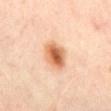Part of a total-body skin-imaging series; this lesion was reviewed on a skin check and was not flagged for biopsy. The patient is a male aged 63–67. The lesion is on the abdomen. This image is a 15 mm lesion crop taken from a total-body photograph. Longest diameter approximately 4 mm. An algorithmic analysis of the crop reported a mean CIELAB color near L≈64 a*≈24 b*≈37, roughly 15 lightness units darker than nearby skin, and a lesion-to-skin contrast of about 9.5 (normalized; higher = more distinct).